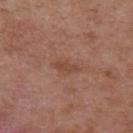{"biopsy_status": "not biopsied; imaged during a skin examination", "patient": {"sex": "female", "age_approx": 40}, "site": "upper back", "lighting": "white-light", "automated_metrics": {"border_irregularity_0_10": 3.0, "color_variation_0_10": 0.5, "peripheral_color_asymmetry": 0.0, "nevus_likeness_0_100": 0}, "image": {"source": "total-body photography crop", "field_of_view_mm": 15}}